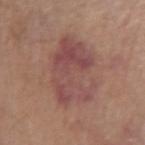notes=imaged on a skin check; not biopsied | patient=female, aged approximately 65 | size=about 7 mm | site=the left forearm | acquisition=~15 mm crop, total-body skin-cancer survey.